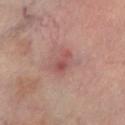Notes:
* biopsy status · imaged on a skin check; not biopsied
* subject · male, aged 68 to 72
* illumination · cross-polarized
* body site · the left lower leg
* imaging modality · total-body-photography crop, ~15 mm field of view